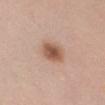Imaged during a routine full-body skin examination; the lesion was not biopsied and no histopathology is available.